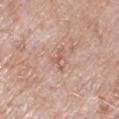The lesion was tiled from a total-body skin photograph and was not biopsied. The lesion's longest dimension is about 3 mm. On the right upper arm. The tile uses white-light illumination. Automated tile analysis of the lesion measured an automated nevus-likeness rating near 0 out of 100 and lesion-presence confidence of about 95/100. The subject is a male aged 63–67. A region of skin cropped from a whole-body photographic capture, roughly 15 mm wide.